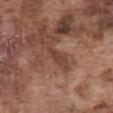Notes:
• biopsy status: no biopsy performed (imaged during a skin exam)
• body site: the front of the torso
• lighting: white-light illumination
• subject: male, approximately 75 years of age
• image source: total-body-photography crop, ~15 mm field of view